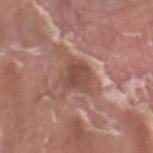Assessment: Part of a total-body skin-imaging series; this lesion was reviewed on a skin check and was not flagged for biopsy. Clinical summary: The lesion is located on the left thigh. A male patient, aged 38–42. About 3.5 mm across. The tile uses white-light illumination. A 15 mm close-up extracted from a 3D total-body photography capture.